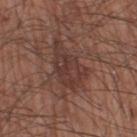The tile uses white-light illumination. The recorded lesion diameter is about 6.5 mm. Automated image analysis of the tile measured a footprint of about 16 mm² and a symmetry-axis asymmetry near 0.35. The software also gave a border-irregularity index near 5.5/10, a color-variation rating of about 3.5/10, and peripheral color asymmetry of about 1. The software also gave a classifier nevus-likeness of about 10/100 and a detector confidence of about 95 out of 100 that the crop contains a lesion. The patient is a male aged approximately 60. The lesion is located on the leg. Cropped from a whole-body photographic skin survey; the tile spans about 15 mm.